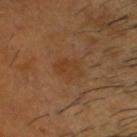Case summary:
* image: total-body-photography crop, ~15 mm field of view
* location: the head or neck
* subject: male, in their 60s